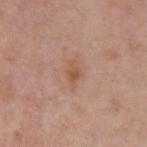notes: total-body-photography surveillance lesion; no biopsy
image-analysis metrics: an area of roughly 4 mm², an eccentricity of roughly 0.75, and two-axis asymmetry of about 0.25; a lesion-to-skin contrast of about 6 (normalized; higher = more distinct); a border-irregularity index near 2.5/10, internal color variation of about 3 on a 0–10 scale, and radial color variation of about 1; an automated nevus-likeness rating near 5 out of 100 and a detector confidence of about 100 out of 100 that the crop contains a lesion
image: ~15 mm tile from a whole-body skin photo
site: the left upper arm
patient: female, aged 58–62